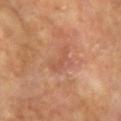follow-up = no biopsy performed (imaged during a skin exam) | anatomic site = the arm | patient = female, aged around 75 | image = ~15 mm tile from a whole-body skin photo.